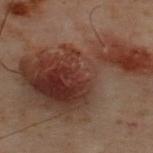Clinical impression: Imaged during a routine full-body skin examination; the lesion was not biopsied and no histopathology is available. Image and clinical context: The recorded lesion diameter is about 14 mm. The patient is a male approximately 50 years of age. This is a cross-polarized tile. A lesion tile, about 15 mm wide, cut from a 3D total-body photograph. From the upper back.